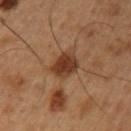Assessment:
Captured during whole-body skin photography for melanoma surveillance; the lesion was not biopsied.
Image and clinical context:
Approximately 4 mm at its widest. The subject is a male about 50 years old. Cropped from a whole-body photographic skin survey; the tile spans about 15 mm. Located on the left upper arm.